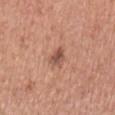Assessment: Part of a total-body skin-imaging series; this lesion was reviewed on a skin check and was not flagged for biopsy.